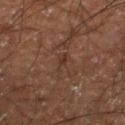The subject is a male aged around 60.
Imaged with cross-polarized lighting.
A 15 mm close-up extracted from a 3D total-body photography capture.
Longest diameter approximately 3 mm.
The lesion is on the right lower leg.